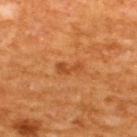The lesion was photographed on a routine skin check and not biopsied; there is no pathology result.